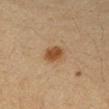Impression:
No biopsy was performed on this lesion — it was imaged during a full skin examination and was not determined to be concerning.
Acquisition and patient details:
The lesion is on the right forearm. The total-body-photography lesion software estimated a mean CIELAB color near L≈41 a*≈17 b*≈31, a lesion–skin lightness drop of about 10, and a normalized lesion–skin contrast near 8.5. The analysis additionally found a color-variation rating of about 2/10 and peripheral color asymmetry of about 0.5. The analysis additionally found a detector confidence of about 100 out of 100 that the crop contains a lesion. Captured under cross-polarized illumination. Cropped from a whole-body photographic skin survey; the tile spans about 15 mm. Measured at roughly 2.5 mm in maximum diameter. A female patient, aged around 40.